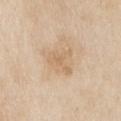No biopsy was performed on this lesion — it was imaged during a full skin examination and was not determined to be concerning.
Automated tile analysis of the lesion measured a footprint of about 5.5 mm², a shape eccentricity near 0.75, and a symmetry-axis asymmetry near 0.6. It also reported a lesion color around L≈66 a*≈15 b*≈35 in CIELAB and a normalized border contrast of about 5. And it measured a nevus-likeness score of about 0/100 and a lesion-detection confidence of about 100/100.
Approximately 3.5 mm at its widest.
Cropped from a total-body skin-imaging series; the visible field is about 15 mm.
Located on the left upper arm.
The patient is a male about 80 years old.